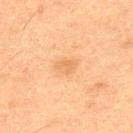Notes:
– workup · catalogued during a skin exam; not biopsied
– subject · male, aged 48 to 52
– lighting · cross-polarized
– image source · 15 mm crop, total-body photography
– site · the back
– TBP lesion metrics · a lesion color around L≈54 a*≈19 b*≈36 in CIELAB, about 6 CIELAB-L* units darker than the surrounding skin, and a normalized border contrast of about 5; internal color variation of about 1 on a 0–10 scale and radial color variation of about 0.5
– lesion size · ≈3 mm